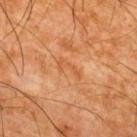  biopsy_status: not biopsied; imaged during a skin examination
  image:
    source: total-body photography crop
    field_of_view_mm: 15
  site: upper back
  automated_metrics:
    cielab_L: 45
    cielab_a: 22
    cielab_b: 35
    vs_skin_darker_L: 5.0
    vs_skin_contrast_norm: 4.5
    border_irregularity_0_10: 7.0
    color_variation_0_10: 0.0
    peripheral_color_asymmetry: 0.0
    lesion_detection_confidence_0_100: 100
  patient:
    sex: male
    age_approx: 65